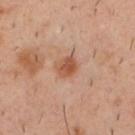A 15 mm close-up tile from a total-body photography series done for melanoma screening.
A male subject approximately 40 years of age.
Measured at roughly 2.5 mm in maximum diameter.
On the upper back.
Captured under cross-polarized illumination.
An algorithmic analysis of the crop reported a mean CIELAB color near L≈52 a*≈24 b*≈32, roughly 10 lightness units darker than nearby skin, and a lesion-to-skin contrast of about 7.5 (normalized; higher = more distinct). The software also gave a classifier nevus-likeness of about 90/100.